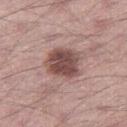biopsy status = catalogued during a skin exam; not biopsied.